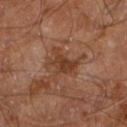{"biopsy_status": "not biopsied; imaged during a skin examination", "lighting": "cross-polarized", "automated_metrics": {"area_mm2_approx": 6.0, "eccentricity": 0.8, "shape_asymmetry": 0.4, "cielab_L": 37, "cielab_a": 21, "cielab_b": 30, "vs_skin_darker_L": 8.0, "vs_skin_contrast_norm": 7.5, "nevus_likeness_0_100": 0, "lesion_detection_confidence_0_100": 100}, "lesion_size": {"long_diameter_mm_approx": 4.0}, "site": "right leg", "image": {"source": "total-body photography crop", "field_of_view_mm": 15}, "patient": {"sex": "male", "age_approx": 60}}